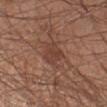Part of a total-body skin-imaging series; this lesion was reviewed on a skin check and was not flagged for biopsy. A close-up tile cropped from a whole-body skin photograph, about 15 mm across. A male subject, aged 18–22. The lesion is located on the right forearm. Longest diameter approximately 3 mm.